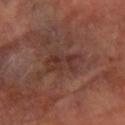biopsy_status: not biopsied; imaged during a skin examination
automated_metrics:
  area_mm2_approx: 9.0
  eccentricity: 0.85
  shape_asymmetry: 0.3
  cielab_L: 34
  cielab_a: 21
  cielab_b: 24
  vs_skin_darker_L: 7.0
site: arm
lesion_size:
  long_diameter_mm_approx: 4.5
image:
  source: total-body photography crop
  field_of_view_mm: 15
lighting: cross-polarized
patient:
  sex: female
  age_approx: 60